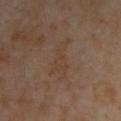| feature | finding |
|---|---|
| notes | no biopsy performed (imaged during a skin exam) |
| image | ~15 mm crop, total-body skin-cancer survey |
| lighting | cross-polarized illumination |
| subject | male, aged 53 to 57 |
| location | the upper back |